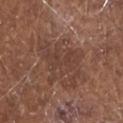notes: imaged on a skin check; not biopsied
size: about 5 mm
image source: total-body-photography crop, ~15 mm field of view
patient: male, roughly 80 years of age
lighting: white-light
site: the head or neck